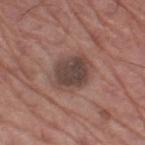Assessment:
Imaged during a routine full-body skin examination; the lesion was not biopsied and no histopathology is available.
Background:
A male subject approximately 70 years of age. Imaged with white-light lighting. Cropped from a total-body skin-imaging series; the visible field is about 15 mm. The recorded lesion diameter is about 4 mm. The lesion is on the left thigh.